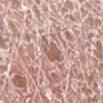Clinical impression:
Imaged during a routine full-body skin examination; the lesion was not biopsied and no histopathology is available.
Acquisition and patient details:
This is a white-light tile. A male patient roughly 75 years of age. A lesion tile, about 15 mm wide, cut from a 3D total-body photograph. Automated image analysis of the tile measured a mean CIELAB color near L≈59 a*≈20 b*≈25, about 10 CIELAB-L* units darker than the surrounding skin, and a normalized lesion–skin contrast near 7. From the leg.